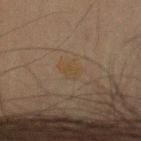biopsy status: total-body-photography surveillance lesion; no biopsy
body site: the arm
subject: male, in their mid- to late 60s
image: total-body-photography crop, ~15 mm field of view